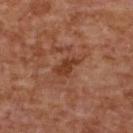Clinical impression: No biopsy was performed on this lesion — it was imaged during a full skin examination and was not determined to be concerning. Image and clinical context: Located on the upper back. Approximately 3 mm at its widest. The lesion-visualizer software estimated an automated nevus-likeness rating near 5 out of 100 and a detector confidence of about 100 out of 100 that the crop contains a lesion. Imaged with cross-polarized lighting. A female subject aged 58 to 62. A region of skin cropped from a whole-body photographic capture, roughly 15 mm wide.